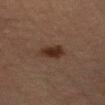follow-up: catalogued during a skin exam; not biopsied
tile lighting: cross-polarized illumination
anatomic site: the left upper arm
imaging modality: ~15 mm tile from a whole-body skin photo
lesion diameter: ≈3 mm
TBP lesion metrics: a lesion–skin lightness drop of about 8 and a lesion-to-skin contrast of about 10 (normalized; higher = more distinct); an automated nevus-likeness rating near 90 out of 100 and a lesion-detection confidence of about 100/100
patient: female, approximately 55 years of age